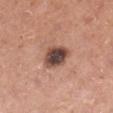follow-up = total-body-photography surveillance lesion; no biopsy
body site = the leg
imaging modality = total-body-photography crop, ~15 mm field of view
patient = male, aged around 55